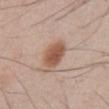Recorded during total-body skin imaging; not selected for excision or biopsy. On the front of the torso. Imaged with white-light lighting. A male patient approximately 45 years of age. This image is a 15 mm lesion crop taken from a total-body photograph. The recorded lesion diameter is about 4 mm. Automated image analysis of the tile measured an eccentricity of roughly 0.7. The analysis additionally found a border-irregularity rating of about 1.5/10, internal color variation of about 3.5 on a 0–10 scale, and radial color variation of about 1. And it measured an automated nevus-likeness rating near 100 out of 100 and lesion-presence confidence of about 100/100.